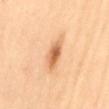Q: What did automated image analysis measure?
A: a classifier nevus-likeness of about 95/100 and a detector confidence of about 100 out of 100 that the crop contains a lesion
Q: What is the anatomic site?
A: the mid back
Q: What is the imaging modality?
A: 15 mm crop, total-body photography
Q: Who is the patient?
A: female, aged approximately 60